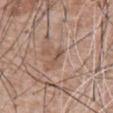Image and clinical context:
Located on the chest. The tile uses white-light illumination. A 15 mm crop from a total-body photograph taken for skin-cancer surveillance. The subject is a male aged approximately 60. Automated tile analysis of the lesion measured a nevus-likeness score of about 0/100 and a lesion-detection confidence of about 60/100. Measured at roughly 2.5 mm in maximum diameter.